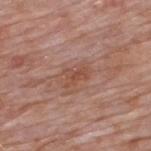Q: Is there a histopathology result?
A: catalogued during a skin exam; not biopsied
Q: Lesion location?
A: the upper back
Q: What kind of image is this?
A: 15 mm crop, total-body photography
Q: Who is the patient?
A: male, in their 60s
Q: How large is the lesion?
A: ~3 mm (longest diameter)